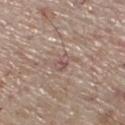workup: catalogued during a skin exam; not biopsied | size: ~2.5 mm (longest diameter) | image-analysis metrics: an area of roughly 4 mm² and a symmetry-axis asymmetry near 0.4; an average lesion color of about L≈53 a*≈18 b*≈21 (CIELAB), roughly 8 lightness units darker than nearby skin, and a lesion-to-skin contrast of about 5.5 (normalized; higher = more distinct); border irregularity of about 4 on a 0–10 scale, internal color variation of about 3.5 on a 0–10 scale, and a peripheral color-asymmetry measure near 1 | acquisition: ~15 mm crop, total-body skin-cancer survey | anatomic site: the left lower leg | patient: male, about 70 years old | illumination: white-light.